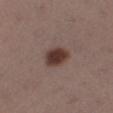Impression:
Recorded during total-body skin imaging; not selected for excision or biopsy.
Acquisition and patient details:
A region of skin cropped from a whole-body photographic capture, roughly 15 mm wide. The lesion is located on the left lower leg. The subject is a female aged around 30.